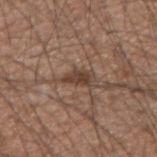  patient:
    sex: male
    age_approx: 35
  automated_metrics:
    area_mm2_approx: 3.5
    shape_asymmetry: 0.4
    peripheral_color_asymmetry: 0.5
  lesion_size:
    long_diameter_mm_approx: 3.0
  site: right forearm
  image:
    source: total-body photography crop
    field_of_view_mm: 15